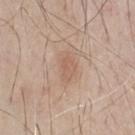site = the chest | imaging modality = ~15 mm crop, total-body skin-cancer survey | subject = male, about 65 years old.